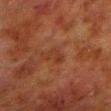The lesion was photographed on a routine skin check and not biopsied; there is no pathology result.
The lesion is located on the left lower leg.
A male patient approximately 80 years of age.
Imaged with cross-polarized lighting.
A region of skin cropped from a whole-body photographic capture, roughly 15 mm wide.